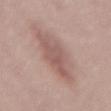biopsy_status: not biopsied; imaged during a skin examination
image:
  source: total-body photography crop
  field_of_view_mm: 15
site: mid back
patient:
  sex: female
  age_approx: 50
lighting: white-light